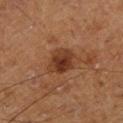notes: catalogued during a skin exam; not biopsied
subject: male, in their mid- to late 70s
lesion diameter: about 3.5 mm
site: the left lower leg
lighting: cross-polarized illumination
acquisition: total-body-photography crop, ~15 mm field of view
automated metrics: peripheral color asymmetry of about 1; an automated nevus-likeness rating near 80 out of 100 and a lesion-detection confidence of about 100/100